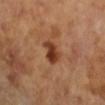No biopsy was performed on this lesion — it was imaged during a full skin examination and was not determined to be concerning. A 15 mm crop from a total-body photograph taken for skin-cancer surveillance. Captured under cross-polarized illumination. Measured at roughly 3 mm in maximum diameter. A female patient about 65 years old. The lesion is on the left arm. Automated tile analysis of the lesion measured border irregularity of about 3 on a 0–10 scale and internal color variation of about 4 on a 0–10 scale.